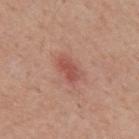The lesion was photographed on a routine skin check and not biopsied; there is no pathology result. A male patient, approximately 55 years of age. The lesion is located on the mid back. Cropped from a total-body skin-imaging series; the visible field is about 15 mm. Measured at roughly 3.5 mm in maximum diameter. Imaged with white-light lighting.